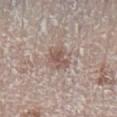automated_metrics:
  border_irregularity_0_10: 3.5
  peripheral_color_asymmetry: 0.5
lesion_size:
  long_diameter_mm_approx: 3.0
patient:
  sex: male
  age_approx: 60
site: left lower leg
lighting: white-light
image:
  source: total-body photography crop
  field_of_view_mm: 15